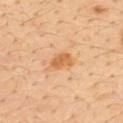Q: Was this lesion biopsied?
A: no biopsy performed (imaged during a skin exam)
Q: Illumination type?
A: cross-polarized
Q: What is the imaging modality?
A: total-body-photography crop, ~15 mm field of view
Q: Who is the patient?
A: male, aged approximately 35
Q: How large is the lesion?
A: ~2.5 mm (longest diameter)
Q: Automated lesion metrics?
A: a lesion area of about 4.5 mm² and a shape eccentricity near 0.65; roughly 9 lightness units darker than nearby skin and a normalized border contrast of about 7.5; a nevus-likeness score of about 55/100 and a lesion-detection confidence of about 100/100
Q: Where on the body is the lesion?
A: the upper back